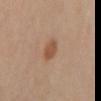Imaged during a routine full-body skin examination; the lesion was not biopsied and no histopathology is available.
Imaged with cross-polarized lighting.
This image is a 15 mm lesion crop taken from a total-body photograph.
A male patient, roughly 65 years of age.
Automated image analysis of the tile measured a classifier nevus-likeness of about 95/100 and a detector confidence of about 100 out of 100 that the crop contains a lesion.
The lesion is located on the mid back.
About 3.5 mm across.